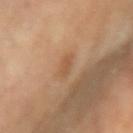Clinical impression: This lesion was catalogued during total-body skin photography and was not selected for biopsy. Acquisition and patient details: A region of skin cropped from a whole-body photographic capture, roughly 15 mm wide. The lesion is on the right upper arm. The subject is a female aged 63–67. Imaged with cross-polarized lighting.